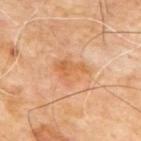workup: no biopsy performed (imaged during a skin exam); patient: male, about 65 years old; image source: total-body-photography crop, ~15 mm field of view; site: the back.